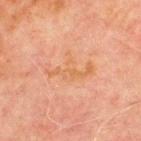biopsy_status: not biopsied; imaged during a skin examination
automated_metrics:
  area_mm2_approx: 9.0
  eccentricity: 0.85
  shape_asymmetry: 0.5
  cielab_L: 53
  cielab_a: 21
  cielab_b: 34
  vs_skin_darker_L: 4.0
  vs_skin_contrast_norm: 5.5
  border_irregularity_0_10: 8.5
  color_variation_0_10: 2.0
  lesion_detection_confidence_0_100: 100
patient:
  sex: male
  age_approx: 70
site: chest
lighting: cross-polarized
image:
  source: total-body photography crop
  field_of_view_mm: 15